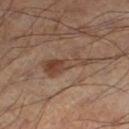<lesion>
<biopsy_status>not biopsied; imaged during a skin examination</biopsy_status>
<site>left lower leg</site>
<image>
  <source>total-body photography crop</source>
  <field_of_view_mm>15</field_of_view_mm>
</image>
<patient>
  <sex>male</sex>
  <age_approx>55</age_approx>
</patient>
<lesion_size>
  <long_diameter_mm_approx>6.5</long_diameter_mm_approx>
</lesion_size>
</lesion>